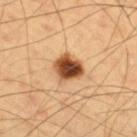Clinical impression: Recorded during total-body skin imaging; not selected for excision or biopsy.